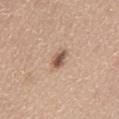Q: Is there a histopathology result?
A: total-body-photography surveillance lesion; no biopsy
Q: How was this image acquired?
A: total-body-photography crop, ~15 mm field of view
Q: Lesion size?
A: ≈2.5 mm
Q: Where on the body is the lesion?
A: the back
Q: Who is the patient?
A: male, aged 63 to 67
Q: How was the tile lit?
A: white-light